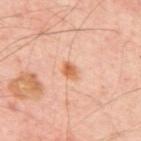workup: total-body-photography surveillance lesion; no biopsy | size: ~2.5 mm (longest diameter) | automated lesion analysis: a lesion area of about 3.5 mm²; a lesion color around L≈64 a*≈25 b*≈37 in CIELAB, about 10 CIELAB-L* units darker than the surrounding skin, and a normalized border contrast of about 7.5; a within-lesion color-variation index near 4.5/10; a nevus-likeness score of about 95/100 | tile lighting: cross-polarized illumination | anatomic site: the mid back | image source: ~15 mm crop, total-body skin-cancer survey | subject: roughly 55 years of age.